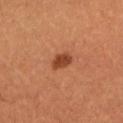notes — imaged on a skin check; not biopsied | subject — female, roughly 30 years of age | image-analysis metrics — an area of roughly 4.5 mm², a shape eccentricity near 0.8, and two-axis asymmetry of about 0.3; a border-irregularity index near 2.5/10, internal color variation of about 1.5 on a 0–10 scale, and radial color variation of about 0.5; a classifier nevus-likeness of about 95/100 and a lesion-detection confidence of about 100/100 | size — about 3 mm | anatomic site — the left lower leg | tile lighting — cross-polarized | imaging modality — 15 mm crop, total-body photography.